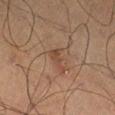Automated image analysis of the tile measured a lesion area of about 3.5 mm², an eccentricity of roughly 0.85, and a symmetry-axis asymmetry near 0.6. And it measured a border-irregularity rating of about 6.5/10, internal color variation of about 0.5 on a 0–10 scale, and peripheral color asymmetry of about 0.
Longest diameter approximately 3 mm.
A male subject, approximately 70 years of age.
A 15 mm close-up extracted from a 3D total-body photography capture.
From the left thigh.
Captured under cross-polarized illumination.